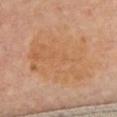This lesion was catalogued during total-body skin photography and was not selected for biopsy. A female subject, about 60 years old. Located on the upper back. A 15 mm close-up extracted from a 3D total-body photography capture.